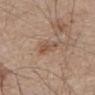The lesion was photographed on a routine skin check and not biopsied; there is no pathology result. A 15 mm close-up tile from a total-body photography series done for melanoma screening. About 3 mm across. Located on the abdomen. The patient is a male in their 80s. The tile uses white-light illumination.